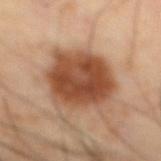Imaged during a routine full-body skin examination; the lesion was not biopsied and no histopathology is available.
The lesion is located on the abdomen.
The subject is a male aged 63 to 67.
This image is a 15 mm lesion crop taken from a total-body photograph.